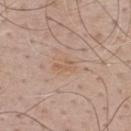notes: imaged on a skin check; not biopsied
imaging modality: ~15 mm tile from a whole-body skin photo
site: the upper back
patient: male, in their mid-40s